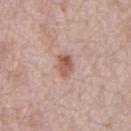follow-up: no biopsy performed (imaged during a skin exam)
subject: male, aged 68 to 72
image source: ~15 mm tile from a whole-body skin photo
site: the front of the torso
diameter: ~2.5 mm (longest diameter)